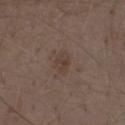Q: Was this lesion biopsied?
A: total-body-photography surveillance lesion; no biopsy
Q: What is the lesion's diameter?
A: ≈3 mm
Q: Where on the body is the lesion?
A: the abdomen
Q: Who is the patient?
A: male, aged around 70
Q: How was the tile lit?
A: white-light
Q: How was this image acquired?
A: ~15 mm tile from a whole-body skin photo